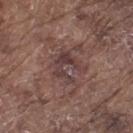| field | value |
|---|---|
| follow-up | catalogued during a skin exam; not biopsied |
| patient | male, in their mid-70s |
| lesion diameter | ≈4 mm |
| illumination | white-light |
| imaging modality | ~15 mm crop, total-body skin-cancer survey |
| site | the left thigh |